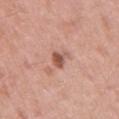| key | value |
|---|---|
| biopsy status | no biopsy performed (imaged during a skin exam) |
| image source | ~15 mm tile from a whole-body skin photo |
| location | the back |
| subject | male, aged 53–57 |